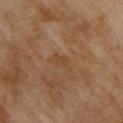This lesion was catalogued during total-body skin photography and was not selected for biopsy. A close-up tile cropped from a whole-body skin photograph, about 15 mm across. Measured at roughly 3 mm in maximum diameter. On the back. Captured under cross-polarized illumination. A male patient, approximately 70 years of age.